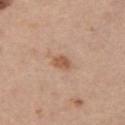biopsy status = catalogued during a skin exam; not biopsied | site = the chest | tile lighting = white-light illumination | imaging modality = 15 mm crop, total-body photography | patient = female, about 45 years old.